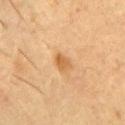{
  "biopsy_status": "not biopsied; imaged during a skin examination",
  "patient": {
    "sex": "male",
    "age_approx": 60
  },
  "lighting": "cross-polarized",
  "image": {
    "source": "total-body photography crop",
    "field_of_view_mm": 15
  },
  "automated_metrics": {
    "color_variation_0_10": 2.0,
    "peripheral_color_asymmetry": 1.0,
    "nevus_likeness_0_100": 50,
    "lesion_detection_confidence_0_100": 100
  },
  "site": "chest",
  "lesion_size": {
    "long_diameter_mm_approx": 2.5
  }
}